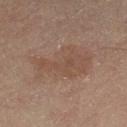No biopsy was performed on this lesion — it was imaged during a full skin examination and was not determined to be concerning.
The patient is a male aged around 55.
Longest diameter approximately 7.5 mm.
This is a cross-polarized tile.
An algorithmic analysis of the crop reported an area of roughly 22 mm² and an outline eccentricity of about 0.85 (0 = round, 1 = elongated). The software also gave a mean CIELAB color near L≈41 a*≈15 b*≈23, about 5 CIELAB-L* units darker than the surrounding skin, and a normalized lesion–skin contrast near 4.5. And it measured a nevus-likeness score of about 0/100 and lesion-presence confidence of about 100/100.
The lesion is on the left thigh.
A 15 mm close-up tile from a total-body photography series done for melanoma screening.